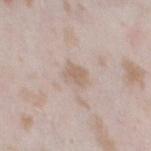| key | value |
|---|---|
| workup | catalogued during a skin exam; not biopsied |
| subject | female, roughly 25 years of age |
| acquisition | ~15 mm tile from a whole-body skin photo |
| lesion size | about 2.5 mm |
| image-analysis metrics | an automated nevus-likeness rating near 0 out of 100 and a lesion-detection confidence of about 100/100 |
| location | the left thigh |